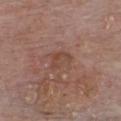<record>
  <biopsy_status>not biopsied; imaged during a skin examination</biopsy_status>
  <patient>
    <sex>male</sex>
    <age_approx>80</age_approx>
  </patient>
  <image>
    <source>total-body photography crop</source>
    <field_of_view_mm>15</field_of_view_mm>
  </image>
  <lighting>white-light</lighting>
  <site>chest</site>
</record>